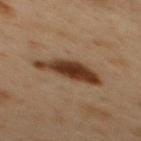Imaged during a routine full-body skin examination; the lesion was not biopsied and no histopathology is available. This is a cross-polarized tile. Cropped from a whole-body photographic skin survey; the tile spans about 15 mm. About 7 mm across. A male subject, aged 48 to 52. From the back.